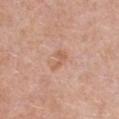follow-up: catalogued during a skin exam; not biopsied
image source: 15 mm crop, total-body photography
patient: female, aged 38 to 42
image-analysis metrics: a footprint of about 3.5 mm², a shape eccentricity near 0.85, and a symmetry-axis asymmetry near 0.5; a mean CIELAB color near L≈60 a*≈23 b*≈32, roughly 7 lightness units darker than nearby skin, and a normalized lesion–skin contrast near 6; a border-irregularity index near 5/10, internal color variation of about 1 on a 0–10 scale, and radial color variation of about 0.5; an automated nevus-likeness rating near 0 out of 100
site: the chest
lesion size: ≈3 mm